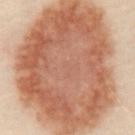• follow-up: catalogued during a skin exam; not biopsied
• patient: male, about 50 years old
• lighting: cross-polarized illumination
• image-analysis metrics: a border-irregularity rating of about 1.5/10, internal color variation of about 5 on a 0–10 scale, and peripheral color asymmetry of about 1.5; an automated nevus-likeness rating near 95 out of 100 and a lesion-detection confidence of about 95/100
• image source: ~15 mm tile from a whole-body skin photo
• body site: the abdomen